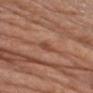Captured during whole-body skin photography for melanoma surveillance; the lesion was not biopsied. Located on the right upper arm. A lesion tile, about 15 mm wide, cut from a 3D total-body photograph. Automated tile analysis of the lesion measured a footprint of about 3 mm², an eccentricity of roughly 0.85, and two-axis asymmetry of about 0.3. It also reported a lesion color around L≈47 a*≈24 b*≈30 in CIELAB and about 8 CIELAB-L* units darker than the surrounding skin. Imaged with white-light lighting. A male patient, in their mid- to late 60s. The recorded lesion diameter is about 2.5 mm.